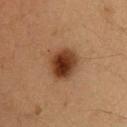lesion diameter = ≈4.5 mm | subject = male, approximately 35 years of age | automated metrics = a border-irregularity index near 2/10, a color-variation rating of about 6.5/10, and a peripheral color-asymmetry measure near 2 | location = the upper back | tile lighting = cross-polarized illumination | image = ~15 mm crop, total-body skin-cancer survey.